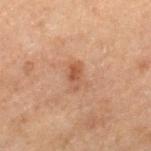Clinical impression:
Imaged during a routine full-body skin examination; the lesion was not biopsied and no histopathology is available.
Clinical summary:
Imaged with cross-polarized lighting. A 15 mm close-up tile from a total-body photography series done for melanoma screening. A male patient, aged approximately 70. On the left thigh. The recorded lesion diameter is about 3 mm. Automated tile analysis of the lesion measured an area of roughly 3.5 mm², an outline eccentricity of about 0.85 (0 = round, 1 = elongated), and two-axis asymmetry of about 0.25. It also reported a normalized border contrast of about 6.5. The analysis additionally found a nevus-likeness score of about 40/100.